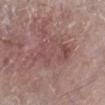No biopsy was performed on this lesion — it was imaged during a full skin examination and was not determined to be concerning.
The lesion is on the left lower leg.
Captured under white-light illumination.
A female subject, aged 73–77.
About 6.5 mm across.
A 15 mm close-up extracted from a 3D total-body photography capture.